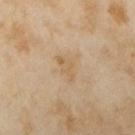This lesion was catalogued during total-body skin photography and was not selected for biopsy.
Approximately 3.5 mm at its widest.
The total-body-photography lesion software estimated a mean CIELAB color near L≈58 a*≈14 b*≈34, roughly 6 lightness units darker than nearby skin, and a normalized lesion–skin contrast near 5.
A female patient in their mid-30s.
Captured under cross-polarized illumination.
On the arm.
A region of skin cropped from a whole-body photographic capture, roughly 15 mm wide.